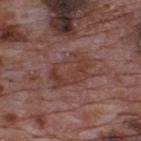Captured during whole-body skin photography for melanoma surveillance; the lesion was not biopsied. Located on the upper back. The patient is a male aged around 70. A 15 mm close-up extracted from a 3D total-body photography capture. The lesion's longest dimension is about 5.5 mm. Automated tile analysis of the lesion measured an automated nevus-likeness rating near 0 out of 100 and a lesion-detection confidence of about 95/100.